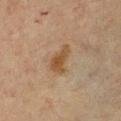Assessment: Part of a total-body skin-imaging series; this lesion was reviewed on a skin check and was not flagged for biopsy. Acquisition and patient details: About 4 mm across. An algorithmic analysis of the crop reported a lesion color around L≈43 a*≈15 b*≈31 in CIELAB, about 8 CIELAB-L* units darker than the surrounding skin, and a normalized border contrast of about 8. The software also gave a border-irregularity rating of about 4/10 and radial color variation of about 0.5. The tile uses cross-polarized illumination. A female subject, aged 63–67. On the chest. A region of skin cropped from a whole-body photographic capture, roughly 15 mm wide.